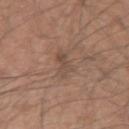biopsy status: no biopsy performed (imaged during a skin exam); patient: male, aged 43–47; image: 15 mm crop, total-body photography; site: the arm; automated metrics: a classifier nevus-likeness of about 0/100 and lesion-presence confidence of about 55/100; size: about 4 mm; illumination: white-light illumination.